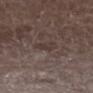<case>
  <biopsy_status>not biopsied; imaged during a skin examination</biopsy_status>
  <patient>
    <sex>male</sex>
    <age_approx>75</age_approx>
  </patient>
  <site>right lower leg</site>
  <lighting>white-light</lighting>
  <image>
    <source>total-body photography crop</source>
    <field_of_view_mm>15</field_of_view_mm>
  </image>
  <lesion_size>
    <long_diameter_mm_approx>2.5</long_diameter_mm_approx>
  </lesion_size>
</case>